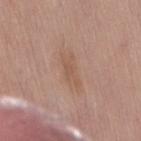notes = catalogued during a skin exam; not biopsied
site = the back
imaging modality = 15 mm crop, total-body photography
patient = female, aged around 70